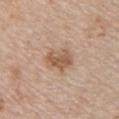<tbp_lesion>
  <biopsy_status>not biopsied; imaged during a skin examination</biopsy_status>
  <image>
    <source>total-body photography crop</source>
    <field_of_view_mm>15</field_of_view_mm>
  </image>
  <site>chest</site>
  <patient>
    <sex>female</sex>
    <age_approx>45</age_approx>
  </patient>
</tbp_lesion>